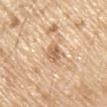biopsy status — catalogued during a skin exam; not biopsied | image — ~15 mm crop, total-body skin-cancer survey | anatomic site — the arm | patient — male, roughly 70 years of age | diameter — about 3 mm.